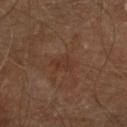Captured during whole-body skin photography for melanoma surveillance; the lesion was not biopsied. Cropped from a whole-body photographic skin survey; the tile spans about 15 mm. Captured under cross-polarized illumination. The lesion-visualizer software estimated a lesion area of about 4 mm². The software also gave a lesion color around L≈33 a*≈18 b*≈27 in CIELAB, about 5 CIELAB-L* units darker than the surrounding skin, and a lesion-to-skin contrast of about 5 (normalized; higher = more distinct). The software also gave a border-irregularity rating of about 4/10, a within-lesion color-variation index near 1/10, and radial color variation of about 0.5. The subject is a male aged approximately 70. The lesion is located on the right lower leg. Measured at roughly 3 mm in maximum diameter.